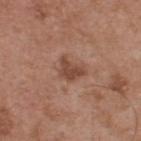{"biopsy_status": "not biopsied; imaged during a skin examination", "site": "upper back", "image": {"source": "total-body photography crop", "field_of_view_mm": 15}, "patient": {"sex": "male", "age_approx": 55}}